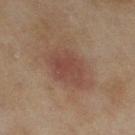No biopsy was performed on this lesion — it was imaged during a full skin examination and was not determined to be concerning.
A female subject, roughly 60 years of age.
The tile uses cross-polarized illumination.
The lesion is located on the left thigh.
About 6.5 mm across.
A lesion tile, about 15 mm wide, cut from a 3D total-body photograph.